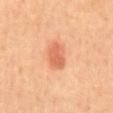Captured during whole-body skin photography for melanoma surveillance; the lesion was not biopsied. A male subject, approximately 65 years of age. The lesion's longest dimension is about 4 mm. The lesion is on the mid back. Automated tile analysis of the lesion measured an average lesion color of about L≈64 a*≈30 b*≈38 (CIELAB) and about 11 CIELAB-L* units darker than the surrounding skin. It also reported a border-irregularity rating of about 2/10 and a within-lesion color-variation index near 3/10. A 15 mm close-up tile from a total-body photography series done for melanoma screening. Imaged with cross-polarized lighting.